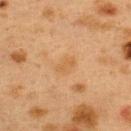biopsy status = total-body-photography surveillance lesion; no biopsy
TBP lesion metrics = an average lesion color of about L≈49 a*≈17 b*≈35 (CIELAB), a lesion–skin lightness drop of about 5, and a normalized border contrast of about 5; a classifier nevus-likeness of about 0/100 and lesion-presence confidence of about 100/100
image source = ~15 mm tile from a whole-body skin photo
anatomic site = the upper back
subject = female, aged 38 to 42